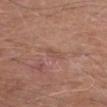Recorded during total-body skin imaging; not selected for excision or biopsy. The tile uses white-light illumination. Cropped from a whole-body photographic skin survey; the tile spans about 15 mm. On the right forearm. A male subject, in their mid- to late 60s.